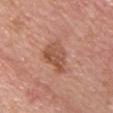biopsy_status: not biopsied; imaged during a skin examination
patient:
  sex: female
  age_approx: 45
lighting: white-light
site: chest
image:
  source: total-body photography crop
  field_of_view_mm: 15
lesion_size:
  long_diameter_mm_approx: 4.0